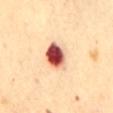workup: total-body-photography surveillance lesion; no biopsy
diameter: ~3.5 mm (longest diameter)
tile lighting: cross-polarized
image: ~15 mm tile from a whole-body skin photo
automated lesion analysis: an area of roughly 8.5 mm², an outline eccentricity of about 0.65 (0 = round, 1 = elongated), and a symmetry-axis asymmetry near 0.2; a classifier nevus-likeness of about 0/100 and lesion-presence confidence of about 100/100
site: the front of the torso
patient: female, aged approximately 65